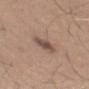Imaged during a routine full-body skin examination; the lesion was not biopsied and no histopathology is available.
A male subject in their mid-60s.
This is a white-light tile.
On the mid back.
A 15 mm crop from a total-body photograph taken for skin-cancer surveillance.
The lesion's longest dimension is about 3.5 mm.